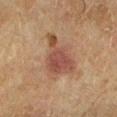Acquisition and patient details:
The lesion-visualizer software estimated an average lesion color of about L≈43 a*≈19 b*≈26 (CIELAB) and a normalized lesion–skin contrast near 7.5. The software also gave border irregularity of about 5.5 on a 0–10 scale, internal color variation of about 4 on a 0–10 scale, and peripheral color asymmetry of about 1. A male subject aged 83 or older. Located on the left lower leg. Cropped from a whole-body photographic skin survey; the tile spans about 15 mm. Captured under cross-polarized illumination. About 6.5 mm across.